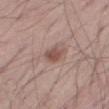Part of a total-body skin-imaging series; this lesion was reviewed on a skin check and was not flagged for biopsy. About 2.5 mm across. Located on the leg. A region of skin cropped from a whole-body photographic capture, roughly 15 mm wide. A male patient, in their mid- to late 50s.